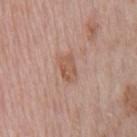follow-up=imaged on a skin check; not biopsied | patient=male, aged around 70 | illumination=white-light illumination | acquisition=15 mm crop, total-body photography | lesion size=about 3 mm | anatomic site=the chest.